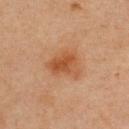Assessment: This lesion was catalogued during total-body skin photography and was not selected for biopsy. Clinical summary: Measured at roughly 4 mm in maximum diameter. A female patient roughly 45 years of age. This image is a 15 mm lesion crop taken from a total-body photograph. Captured under cross-polarized illumination. The lesion is located on the upper back.